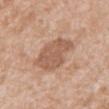Notes:
– follow-up: imaged on a skin check; not biopsied
– lesion size: ~5 mm (longest diameter)
– body site: the abdomen
– image: 15 mm crop, total-body photography
– subject: male, approximately 60 years of age